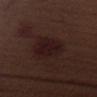On the arm. A male patient aged 68–72. This image is a 15 mm lesion crop taken from a total-body photograph.